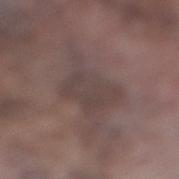workup=imaged on a skin check; not biopsied | lesion size=≈5 mm | image source=15 mm crop, total-body photography | anatomic site=the right lower leg | tile lighting=white-light | patient=male, in their mid-70s.